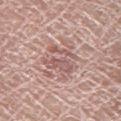{
  "biopsy_status": "not biopsied; imaged during a skin examination",
  "image": {
    "source": "total-body photography crop",
    "field_of_view_mm": 15
  },
  "patient": {
    "sex": "male",
    "age_approx": 75
  },
  "site": "right lower leg",
  "lighting": "white-light",
  "lesion_size": {
    "long_diameter_mm_approx": 4.0
  }
}